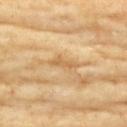biopsy status=total-body-photography surveillance lesion; no biopsy
body site=the front of the torso
image=~15 mm tile from a whole-body skin photo
subject=female, aged 73 to 77
lesion size=~3 mm (longest diameter)
tile lighting=cross-polarized